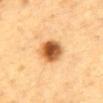A 15 mm close-up extracted from a 3D total-body photography capture. On the abdomen. Approximately 4 mm at its widest. The subject is a male aged approximately 85 or older. An algorithmic analysis of the crop reported a footprint of about 9 mm², a shape eccentricity near 0.6, and two-axis asymmetry of about 0.1. It also reported a nevus-likeness score of about 100/100 and a detector confidence of about 100 out of 100 that the crop contains a lesion.